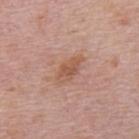Clinical impression:
The lesion was photographed on a routine skin check and not biopsied; there is no pathology result.
Image and clinical context:
From the upper back. The lesion's longest dimension is about 4 mm. A male patient aged 73 to 77. A lesion tile, about 15 mm wide, cut from a 3D total-body photograph.